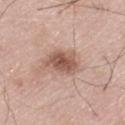biopsy_status: not biopsied; imaged during a skin examination
site: left thigh
lesion_size:
  long_diameter_mm_approx: 4.0
lighting: white-light
patient:
  sex: male
  age_approx: 80
automated_metrics:
  area_mm2_approx: 9.5
  shape_asymmetry: 0.25
  cielab_L: 55
  cielab_a: 20
  cielab_b: 27
  vs_skin_darker_L: 13.0
  nevus_likeness_0_100: 85
  lesion_detection_confidence_0_100: 100
image:
  source: total-body photography crop
  field_of_view_mm: 15